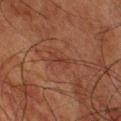Part of a total-body skin-imaging series; this lesion was reviewed on a skin check and was not flagged for biopsy.
A lesion tile, about 15 mm wide, cut from a 3D total-body photograph.
A male patient, aged approximately 60.
From the chest.